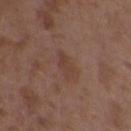follow-up: imaged on a skin check; not biopsied | patient: female, about 35 years old | acquisition: total-body-photography crop, ~15 mm field of view | tile lighting: white-light illumination | TBP lesion metrics: a border-irregularity index near 5.5/10 and internal color variation of about 0 on a 0–10 scale; an automated nevus-likeness rating near 0 out of 100 and lesion-presence confidence of about 100/100 | site: the upper back | size: about 3 mm.